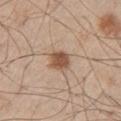Captured during whole-body skin photography for melanoma surveillance; the lesion was not biopsied.
Cropped from a total-body skin-imaging series; the visible field is about 15 mm.
Automated image analysis of the tile measured a classifier nevus-likeness of about 95/100 and lesion-presence confidence of about 100/100.
The subject is a male about 60 years old.
From the left thigh.
This is a white-light tile.